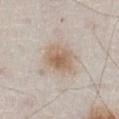No biopsy was performed on this lesion — it was imaged during a full skin examination and was not determined to be concerning.
A male patient aged 78 to 82.
This image is a 15 mm lesion crop taken from a total-body photograph.
The lesion is on the abdomen.
About 4 mm across.